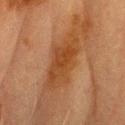Part of a total-body skin-imaging series; this lesion was reviewed on a skin check and was not flagged for biopsy. A 15 mm close-up tile from a total-body photography series done for melanoma screening. This is a cross-polarized tile. Located on the head or neck. A male subject, aged approximately 70. The recorded lesion diameter is about 7.5 mm.